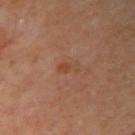Imaged during a routine full-body skin examination; the lesion was not biopsied and no histopathology is available.
The tile uses cross-polarized illumination.
The total-body-photography lesion software estimated a lesion area of about 2.5 mm², a shape eccentricity near 0.9, and a shape-asymmetry score of about 0.35 (0 = symmetric). And it measured a border-irregularity index near 4/10 and radial color variation of about 0. The software also gave a nevus-likeness score of about 0/100.
The recorded lesion diameter is about 2.5 mm.
The subject is a male aged 68–72.
A roughly 15 mm field-of-view crop from a total-body skin photograph.
From the right upper arm.